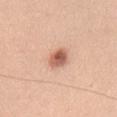notes: imaged on a skin check; not biopsied
image-analysis metrics: a border-irregularity rating of about 2/10, internal color variation of about 6 on a 0–10 scale, and peripheral color asymmetry of about 2; an automated nevus-likeness rating near 100 out of 100 and a detector confidence of about 100 out of 100 that the crop contains a lesion
patient: female, aged around 30
location: the chest
image source: 15 mm crop, total-body photography